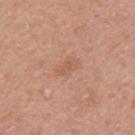notes — total-body-photography surveillance lesion; no biopsy
anatomic site — the arm
lighting — white-light illumination
subject — female, roughly 40 years of age
image source — ~15 mm crop, total-body skin-cancer survey